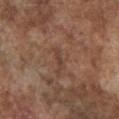<case>
  <biopsy_status>not biopsied; imaged during a skin examination</biopsy_status>
  <patient>
    <sex>male</sex>
    <age_approx>75</age_approx>
  </patient>
  <site>chest</site>
  <image>
    <source>total-body photography crop</source>
    <field_of_view_mm>15</field_of_view_mm>
  </image>
</case>